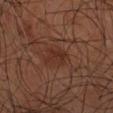{"biopsy_status": "not biopsied; imaged during a skin examination", "image": {"source": "total-body photography crop", "field_of_view_mm": 15}, "lighting": "cross-polarized", "patient": {"sex": "male", "age_approx": 65}, "site": "left upper arm"}